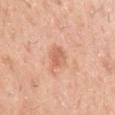Notes:
- biopsy status: imaged on a skin check; not biopsied
- illumination: white-light
- subject: male, roughly 40 years of age
- body site: the left upper arm
- image: 15 mm crop, total-body photography